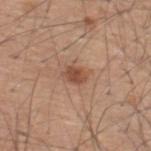<lesion>
  <biopsy_status>not biopsied; imaged during a skin examination</biopsy_status>
  <site>upper back</site>
  <image>
    <source>total-body photography crop</source>
    <field_of_view_mm>15</field_of_view_mm>
  </image>
  <patient>
    <sex>male</sex>
    <age_approx>60</age_approx>
  </patient>
</lesion>